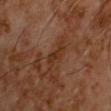Assessment:
Captured during whole-body skin photography for melanoma surveillance; the lesion was not biopsied.
Clinical summary:
The lesion's longest dimension is about 3.5 mm. Imaged with cross-polarized lighting. The subject is a male aged around 60. A roughly 15 mm field-of-view crop from a total-body skin photograph. Located on the chest. Automated tile analysis of the lesion measured a lesion area of about 4 mm², an eccentricity of roughly 0.9, and two-axis asymmetry of about 0.35. The analysis additionally found roughly 7 lightness units darker than nearby skin and a lesion-to-skin contrast of about 7.5 (normalized; higher = more distinct). It also reported border irregularity of about 4.5 on a 0–10 scale, a within-lesion color-variation index near 1/10, and a peripheral color-asymmetry measure near 0.